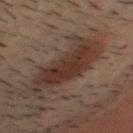Background: On the head or neck. This image is a 15 mm lesion crop taken from a total-body photograph. A male patient, aged approximately 30. Approximately 7.5 mm at its widest.